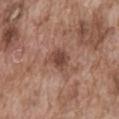Impression: The lesion was tiled from a total-body skin photograph and was not biopsied. Acquisition and patient details: On the abdomen. The tile uses white-light illumination. The total-body-photography lesion software estimated a normalized lesion–skin contrast near 8. And it measured a nevus-likeness score of about 20/100 and a lesion-detection confidence of about 100/100. This image is a 15 mm lesion crop taken from a total-body photograph. A male patient, in their mid-70s. About 2.5 mm across.